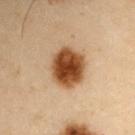Q: Was a biopsy performed?
A: total-body-photography surveillance lesion; no biopsy
Q: Lesion size?
A: ~5 mm (longest diameter)
Q: Illumination type?
A: cross-polarized
Q: Lesion location?
A: the left upper arm
Q: What kind of image is this?
A: ~15 mm crop, total-body skin-cancer survey
Q: What did automated image analysis measure?
A: a footprint of about 15 mm², a shape eccentricity near 0.55, and a shape-asymmetry score of about 0.1 (0 = symmetric); a mean CIELAB color near L≈38 a*≈19 b*≈31, a lesion–skin lightness drop of about 17, and a lesion-to-skin contrast of about 14 (normalized; higher = more distinct); a detector confidence of about 100 out of 100 that the crop contains a lesion
Q: Patient demographics?
A: male, aged 53–57